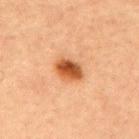Assessment:
Part of a total-body skin-imaging series; this lesion was reviewed on a skin check and was not flagged for biopsy.
Image and clinical context:
On the upper back. Cropped from a total-body skin-imaging series; the visible field is about 15 mm. Approximately 3.5 mm at its widest. A female subject roughly 60 years of age. Imaged with cross-polarized lighting.